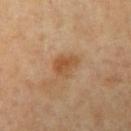biopsy status: catalogued during a skin exam; not biopsied | subject: male, roughly 45 years of age | imaging modality: ~15 mm crop, total-body skin-cancer survey | site: the left upper arm.